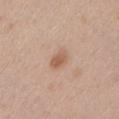follow-up: catalogued during a skin exam; not biopsied
image: 15 mm crop, total-body photography
patient: male, aged around 50
site: the left upper arm
diameter: about 2.5 mm